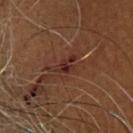The lesion was tiled from a total-body skin photograph and was not biopsied.
The tile uses cross-polarized illumination.
The patient is a female about 80 years old.
The lesion's longest dimension is about 3.5 mm.
The lesion is on the head or neck.
A 15 mm close-up extracted from a 3D total-body photography capture.